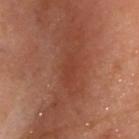Q: What are the patient's age and sex?
A: female, aged around 60
Q: What kind of image is this?
A: ~15 mm tile from a whole-body skin photo
Q: Lesion location?
A: the head or neck
Q: How large is the lesion?
A: ≈3 mm